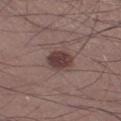Assessment: Imaged during a routine full-body skin examination; the lesion was not biopsied and no histopathology is available. Image and clinical context: Cropped from a total-body skin-imaging series; the visible field is about 15 mm. On the left thigh. Approximately 3.5 mm at its widest. A male subject, aged 53 to 57. The lesion-visualizer software estimated an area of roughly 7.5 mm². It also reported a lesion color around L≈39 a*≈18 b*≈18 in CIELAB, a lesion–skin lightness drop of about 12, and a lesion-to-skin contrast of about 9.5 (normalized; higher = more distinct). The analysis additionally found peripheral color asymmetry of about 1. The software also gave a nevus-likeness score of about 85/100 and a lesion-detection confidence of about 100/100. This is a white-light tile.